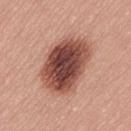No biopsy was performed on this lesion — it was imaged during a full skin examination and was not determined to be concerning. From the lower back. A 15 mm close-up tile from a total-body photography series done for melanoma screening. The tile uses white-light illumination. Approximately 7 mm at its widest. A male subject aged 53–57.